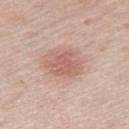<case>
  <biopsy_status>not biopsied; imaged during a skin examination</biopsy_status>
  <site>right thigh</site>
  <patient>
    <sex>female</sex>
    <age_approx>60</age_approx>
  </patient>
  <lesion_size>
    <long_diameter_mm_approx>4.5</long_diameter_mm_approx>
  </lesion_size>
  <image>
    <source>total-body photography crop</source>
    <field_of_view_mm>15</field_of_view_mm>
  </image>
</case>